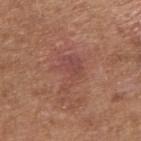Q: Was a biopsy performed?
A: imaged on a skin check; not biopsied
Q: Who is the patient?
A: male, aged around 65
Q: Where on the body is the lesion?
A: the right upper arm
Q: How was this image acquired?
A: total-body-photography crop, ~15 mm field of view